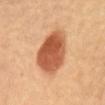Captured during whole-body skin photography for melanoma surveillance; the lesion was not biopsied.
A 15 mm crop from a total-body photograph taken for skin-cancer surveillance.
The lesion is on the chest.
A female subject, aged 58 to 62.